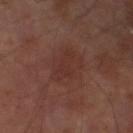Assessment: The lesion was tiled from a total-body skin photograph and was not biopsied. Clinical summary: Longest diameter approximately 5 mm. The lesion-visualizer software estimated an automated nevus-likeness rating near 0 out of 100 and a lesion-detection confidence of about 100/100. A region of skin cropped from a whole-body photographic capture, roughly 15 mm wide. A male subject roughly 70 years of age. Located on the left lower leg. The tile uses cross-polarized illumination.